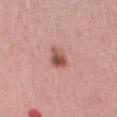Q: Was a biopsy performed?
A: total-body-photography surveillance lesion; no biopsy
Q: Lesion location?
A: the left thigh
Q: What is the imaging modality?
A: ~15 mm tile from a whole-body skin photo
Q: Patient demographics?
A: female, roughly 55 years of age